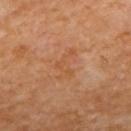Recorded during total-body skin imaging; not selected for excision or biopsy. On the upper back. The lesion-visualizer software estimated a mean CIELAB color near L≈51 a*≈23 b*≈37 and a lesion–skin lightness drop of about 5. And it measured a border-irregularity rating of about 8/10, a color-variation rating of about 1.5/10, and peripheral color asymmetry of about 0. The recorded lesion diameter is about 3.5 mm. A region of skin cropped from a whole-body photographic capture, roughly 15 mm wide. A female patient, aged around 50. The tile uses cross-polarized illumination.